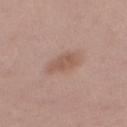No biopsy was performed on this lesion — it was imaged during a full skin examination and was not determined to be concerning. A region of skin cropped from a whole-body photographic capture, roughly 15 mm wide. A female subject aged 38–42. From the arm.